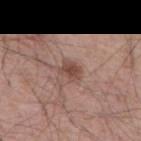Acquisition and patient details: Imaged with white-light lighting. Approximately 2.5 mm at its widest. A region of skin cropped from a whole-body photographic capture, roughly 15 mm wide. A male subject aged 53 to 57. From the mid back.